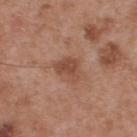Q: How was this image acquired?
A: ~15 mm crop, total-body skin-cancer survey
Q: What did automated image analysis measure?
A: a footprint of about 6.5 mm², a shape eccentricity near 0.65, and two-axis asymmetry of about 0.4; a border-irregularity rating of about 4/10, internal color variation of about 3 on a 0–10 scale, and peripheral color asymmetry of about 1
Q: What is the lesion's diameter?
A: ~3.5 mm (longest diameter)
Q: Lesion location?
A: the upper back
Q: Patient demographics?
A: male, approximately 55 years of age
Q: How was the tile lit?
A: white-light illumination